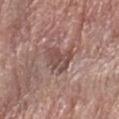The lesion is on the left forearm. Approximately 4 mm at its widest. A male subject aged 73 to 77. The total-body-photography lesion software estimated an outline eccentricity of about 0.75 (0 = round, 1 = elongated) and a shape-asymmetry score of about 0.4 (0 = symmetric). The analysis additionally found a border-irregularity index near 4.5/10, a color-variation rating of about 4/10, and radial color variation of about 1.5. Imaged with white-light lighting. A close-up tile cropped from a whole-body skin photograph, about 15 mm across.